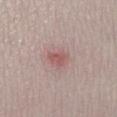follow-up: imaged on a skin check; not biopsied | anatomic site: the left lower leg | automated lesion analysis: an area of roughly 4 mm², an outline eccentricity of about 0.6 (0 = round, 1 = elongated), and two-axis asymmetry of about 0.35; a nevus-likeness score of about 5/100 and a lesion-detection confidence of about 100/100 | lighting: white-light | patient: female, aged approximately 30 | image: ~15 mm tile from a whole-body skin photo | diameter: about 2.5 mm.